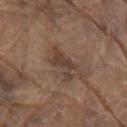<lesion>
<biopsy_status>not biopsied; imaged during a skin examination</biopsy_status>
<lesion_size>
  <long_diameter_mm_approx>4.5</long_diameter_mm_approx>
</lesion_size>
<patient>
  <sex>male</sex>
  <age_approx>80</age_approx>
</patient>
<site>right upper arm</site>
<automated_metrics>
  <nevus_likeness_0_100>10</nevus_likeness_0_100>
  <lesion_detection_confidence_0_100>95</lesion_detection_confidence_0_100>
</automated_metrics>
<lighting>white-light</lighting>
<image>
  <source>total-body photography crop</source>
  <field_of_view_mm>15</field_of_view_mm>
</image>
</lesion>